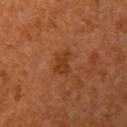Q: Was a biopsy performed?
A: catalogued during a skin exam; not biopsied
Q: Automated lesion metrics?
A: an area of roughly 4 mm², a shape eccentricity near 0.8, and two-axis asymmetry of about 0.45; a lesion color around L≈29 a*≈21 b*≈31 in CIELAB; a classifier nevus-likeness of about 0/100
Q: Lesion size?
A: ~2.5 mm (longest diameter)
Q: How was the tile lit?
A: cross-polarized
Q: Who is the patient?
A: female, roughly 50 years of age
Q: What is the anatomic site?
A: the left upper arm
Q: What is the imaging modality?
A: 15 mm crop, total-body photography